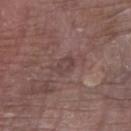No biopsy was performed on this lesion — it was imaged during a full skin examination and was not determined to be concerning.
On the right upper arm.
A male patient in their 80s.
About 2.5 mm across.
Captured under white-light illumination.
Cropped from a whole-body photographic skin survey; the tile spans about 15 mm.